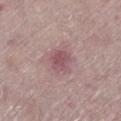Clinical impression: The lesion was tiled from a total-body skin photograph and was not biopsied. Context: From the left lower leg. A female patient, aged approximately 70. A 15 mm close-up tile from a total-body photography series done for melanoma screening.